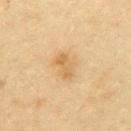Notes:
* follow-up · imaged on a skin check; not biopsied
* acquisition · ~15 mm crop, total-body skin-cancer survey
* body site · the back
* size · about 3 mm
* TBP lesion metrics · a footprint of about 4 mm², an outline eccentricity of about 0.85 (0 = round, 1 = elongated), and a symmetry-axis asymmetry near 0.3; an average lesion color of about L≈56 a*≈15 b*≈39 (CIELAB), a lesion–skin lightness drop of about 8, and a normalized border contrast of about 6.5
* subject · female, approximately 45 years of age
* tile lighting · cross-polarized